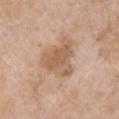Clinical impression:
Part of a total-body skin-imaging series; this lesion was reviewed on a skin check and was not flagged for biopsy.
Image and clinical context:
The lesion is on the right upper arm. A female patient, in their mid- to late 70s. Cropped from a total-body skin-imaging series; the visible field is about 15 mm. The tile uses white-light illumination.